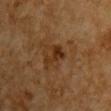Q: Was this lesion biopsied?
A: imaged on a skin check; not biopsied
Q: What is the lesion's diameter?
A: about 3.5 mm
Q: What is the anatomic site?
A: the chest
Q: Who is the patient?
A: male, in their mid-80s or older
Q: What kind of image is this?
A: ~15 mm crop, total-body skin-cancer survey
Q: How was the tile lit?
A: cross-polarized illumination